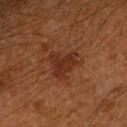Case summary:
• location · the left forearm
• lighting · cross-polarized
• patient · male, aged around 60
• lesion size · about 4.5 mm
• image · ~15 mm tile from a whole-body skin photo